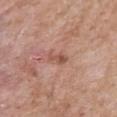- workup: catalogued during a skin exam; not biopsied
- acquisition: 15 mm crop, total-body photography
- lighting: white-light illumination
- patient: male, in their 60s
- size: ≈2.5 mm
- image-analysis metrics: a footprint of about 2.5 mm² and a symmetry-axis asymmetry near 0.4; a within-lesion color-variation index near 0.5/10 and a peripheral color-asymmetry measure near 0
- anatomic site: the front of the torso